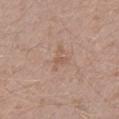Part of a total-body skin-imaging series; this lesion was reviewed on a skin check and was not flagged for biopsy. The recorded lesion diameter is about 3 mm. From the left lower leg. A 15 mm close-up extracted from a 3D total-body photography capture. The subject is a male approximately 45 years of age.